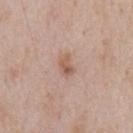A 15 mm close-up tile from a total-body photography series done for melanoma screening. Longest diameter approximately 2.5 mm. The subject is a male aged 58 to 62. The lesion is on the chest. Imaged with white-light lighting.